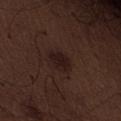<record>
<biopsy_status>not biopsied; imaged during a skin examination</biopsy_status>
<site>abdomen</site>
<automated_metrics>
  <cielab_L>16</cielab_L>
  <cielab_a>15</cielab_a>
  <cielab_b>15</cielab_b>
  <vs_skin_darker_L>7.0</vs_skin_darker_L>
  <vs_skin_contrast_norm>10.0</vs_skin_contrast_norm>
  <nevus_likeness_0_100>45</nevus_likeness_0_100>
</automated_metrics>
<patient>
  <sex>male</sex>
  <age_approx>70</age_approx>
</patient>
<image>
  <source>total-body photography crop</source>
  <field_of_view_mm>15</field_of_view_mm>
</image>
</record>